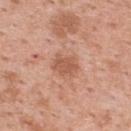notes = catalogued during a skin exam; not biopsied | subject = female, aged 48–52 | lesion diameter = ~3 mm (longest diameter) | imaging modality = total-body-photography crop, ~15 mm field of view | site = the upper back.